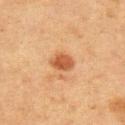This lesion was catalogued during total-body skin photography and was not selected for biopsy.